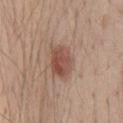| key | value |
|---|---|
| workup | total-body-photography surveillance lesion; no biopsy |
| tile lighting | white-light illumination |
| patient | male, aged around 40 |
| lesion diameter | about 4 mm |
| site | the chest |
| image source | ~15 mm tile from a whole-body skin photo |
| automated metrics | a shape-asymmetry score of about 0.15 (0 = symmetric); a mean CIELAB color near L≈50 a*≈21 b*≈27, a lesion–skin lightness drop of about 11, and a normalized lesion–skin contrast near 8; border irregularity of about 1.5 on a 0–10 scale, internal color variation of about 5 on a 0–10 scale, and peripheral color asymmetry of about 1.5; a detector confidence of about 100 out of 100 that the crop contains a lesion |